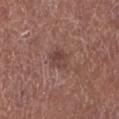notes=no biopsy performed (imaged during a skin exam); lesion size=~2.5 mm (longest diameter); site=the left lower leg; imaging modality=15 mm crop, total-body photography; subject=male, in their mid- to late 60s.